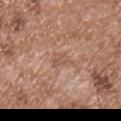This lesion was catalogued during total-body skin photography and was not selected for biopsy. Imaged with white-light lighting. The lesion-visualizer software estimated an area of roughly 3.5 mm², an outline eccentricity of about 0.85 (0 = round, 1 = elongated), and a symmetry-axis asymmetry near 0.4. The analysis additionally found a border-irregularity index near 4/10, internal color variation of about 1 on a 0–10 scale, and a peripheral color-asymmetry measure near 0.5. On the chest. The patient is a male in their mid- to late 50s. This image is a 15 mm lesion crop taken from a total-body photograph. About 3 mm across.